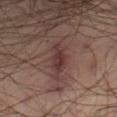Assessment: Imaged during a routine full-body skin examination; the lesion was not biopsied and no histopathology is available. Background: This is a cross-polarized tile. Approximately 5 mm at its widest. A male subject approximately 40 years of age. The lesion is on the right thigh. A 15 mm close-up extracted from a 3D total-body photography capture.